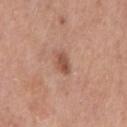biopsy_status: not biopsied; imaged during a skin examination
image:
  source: total-body photography crop
  field_of_view_mm: 15
site: chest
patient:
  sex: male
  age_approx: 75